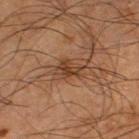Q: Is there a histopathology result?
A: imaged on a skin check; not biopsied
Q: What is the anatomic site?
A: the left thigh
Q: What lighting was used for the tile?
A: cross-polarized
Q: What did automated image analysis measure?
A: a shape eccentricity near 0.75; a lesion color around L≈31 a*≈17 b*≈27 in CIELAB, roughly 8 lightness units darker than nearby skin, and a lesion-to-skin contrast of about 8 (normalized; higher = more distinct); a color-variation rating of about 1/10 and radial color variation of about 0.5
Q: Lesion size?
A: about 2.5 mm
Q: How was this image acquired?
A: ~15 mm crop, total-body skin-cancer survey
Q: Patient demographics?
A: male, in their mid- to late 60s